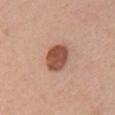Assessment: Imaged during a routine full-body skin examination; the lesion was not biopsied and no histopathology is available. Image and clinical context: A close-up tile cropped from a whole-body skin photograph, about 15 mm across. A female subject aged approximately 25. From the chest.